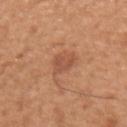Impression:
Recorded during total-body skin imaging; not selected for excision or biopsy.
Background:
This image is a 15 mm lesion crop taken from a total-body photograph. Longest diameter approximately 2.5 mm. This is a white-light tile. The lesion is located on the right upper arm. A male subject, in their mid- to late 50s.